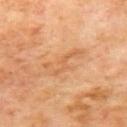Assessment: No biopsy was performed on this lesion — it was imaged during a full skin examination and was not determined to be concerning. Image and clinical context: The lesion is located on the mid back. A male subject aged around 70. The tile uses cross-polarized illumination. A 15 mm close-up extracted from a 3D total-body photography capture.